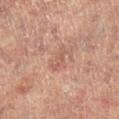A female subject aged approximately 80. This is a cross-polarized tile. A roughly 15 mm field-of-view crop from a total-body skin photograph. The total-body-photography lesion software estimated an area of roughly 3 mm², an outline eccentricity of about 0.9 (0 = round, 1 = elongated), and a shape-asymmetry score of about 0.35 (0 = symmetric). The lesion is located on the left leg.